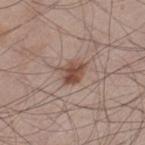Part of a total-body skin-imaging series; this lesion was reviewed on a skin check and was not flagged for biopsy. Imaged with white-light lighting. Approximately 3.5 mm at its widest. The lesion-visualizer software estimated a border-irregularity rating of about 2/10, internal color variation of about 4.5 on a 0–10 scale, and a peripheral color-asymmetry measure near 1.5. The analysis additionally found a nevus-likeness score of about 90/100 and a lesion-detection confidence of about 100/100. From the left thigh. The patient is a male aged 43 to 47. A 15 mm crop from a total-body photograph taken for skin-cancer surveillance.